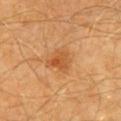The lesion was tiled from a total-body skin photograph and was not biopsied. This image is a 15 mm lesion crop taken from a total-body photograph. The lesion-visualizer software estimated a lesion area of about 6.5 mm², a shape eccentricity near 0.6, and a shape-asymmetry score of about 0.4 (0 = symmetric). The analysis additionally found a border-irregularity index near 3.5/10 and radial color variation of about 1.5. The software also gave an automated nevus-likeness rating near 75 out of 100 and a detector confidence of about 100 out of 100 that the crop contains a lesion. Longest diameter approximately 3 mm. Imaged with cross-polarized lighting. A male subject aged around 60. Located on the front of the torso.